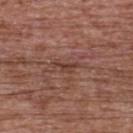Notes:
- biopsy status · total-body-photography surveillance lesion; no biopsy
- diameter · ≈2.5 mm
- body site · the upper back
- patient · female, in their mid-60s
- imaging modality · total-body-photography crop, ~15 mm field of view
- illumination · white-light illumination